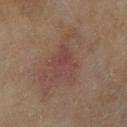Q: Was this lesion biopsied?
A: imaged on a skin check; not biopsied
Q: Where on the body is the lesion?
A: the right lower leg
Q: Lesion size?
A: ≈4 mm
Q: What kind of image is this?
A: ~15 mm tile from a whole-body skin photo
Q: Automated lesion metrics?
A: a lesion area of about 8.5 mm², an outline eccentricity of about 0.6 (0 = round, 1 = elongated), and a symmetry-axis asymmetry near 0.3
Q: Patient demographics?
A: female, about 60 years old
Q: How was the tile lit?
A: cross-polarized illumination